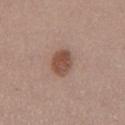• notes — catalogued during a skin exam; not biopsied
• patient — male, aged approximately 55
• acquisition — total-body-photography crop, ~15 mm field of view
• body site — the abdomen
• image-analysis metrics — a lesion area of about 7.5 mm² and a shape-asymmetry score of about 0.15 (0 = symmetric); an average lesion color of about L≈49 a*≈20 b*≈26 (CIELAB) and a normalized lesion–skin contrast near 9; a classifier nevus-likeness of about 85/100